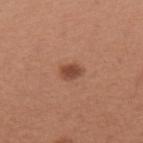notes=imaged on a skin check; not biopsied
subject=female, in their mid- to late 60s
image source=total-body-photography crop, ~15 mm field of view
location=the upper back
diameter=≈2.5 mm